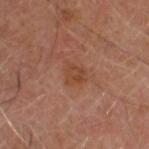Q: Who is the patient?
A: male, aged 63–67
Q: How was this image acquired?
A: ~15 mm tile from a whole-body skin photo
Q: What is the anatomic site?
A: the head or neck